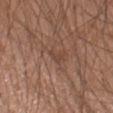<record>
  <biopsy_status>not biopsied; imaged during a skin examination</biopsy_status>
  <patient>
    <sex>male</sex>
    <age_approx>20</age_approx>
  </patient>
  <site>right forearm</site>
  <lesion_size>
    <long_diameter_mm_approx>2.5</long_diameter_mm_approx>
  </lesion_size>
  <image>
    <source>total-body photography crop</source>
    <field_of_view_mm>15</field_of_view_mm>
  </image>
</record>